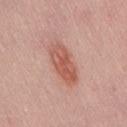A male patient, in their 40s. The lesion is located on the lower back. A 15 mm close-up tile from a total-body photography series done for melanoma screening. The tile uses white-light illumination.